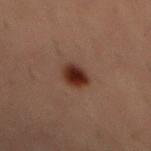follow-up — no biopsy performed (imaged during a skin exam) | automated lesion analysis — a lesion area of about 6 mm², an outline eccentricity of about 0.55 (0 = round, 1 = elongated), and two-axis asymmetry of about 0.15; a lesion color around L≈26 a*≈19 b*≈23 in CIELAB, a lesion–skin lightness drop of about 12, and a lesion-to-skin contrast of about 12 (normalized; higher = more distinct) | lesion diameter — ≈3 mm | patient — male, in their mid-50s | location — the abdomen | image source — 15 mm crop, total-body photography | illumination — cross-polarized illumination.